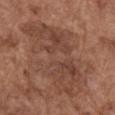* workup: catalogued during a skin exam; not biopsied
* lighting: white-light
* image source: 15 mm crop, total-body photography
* size: about 11.5 mm
* subject: male, in their mid-70s
* location: the arm